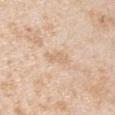{
  "biopsy_status": "not biopsied; imaged during a skin examination",
  "lighting": "white-light",
  "automated_metrics": {
    "area_mm2_approx": 4.0,
    "shape_asymmetry": 0.4,
    "nevus_likeness_0_100": 0,
    "lesion_detection_confidence_0_100": 100
  },
  "site": "right upper arm",
  "image": {
    "source": "total-body photography crop",
    "field_of_view_mm": 15
  },
  "patient": {
    "sex": "male",
    "age_approx": 45
  }
}